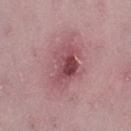No biopsy was performed on this lesion — it was imaged during a full skin examination and was not determined to be concerning. A male subject, about 50 years old. A 15 mm crop from a total-body photograph taken for skin-cancer surveillance. From the left thigh. An algorithmic analysis of the crop reported a footprint of about 17 mm², an eccentricity of roughly 0.7, and a shape-asymmetry score of about 0.25 (0 = symmetric). And it measured a lesion-to-skin contrast of about 7.5 (normalized; higher = more distinct). It also reported an automated nevus-likeness rating near 5 out of 100 and lesion-presence confidence of about 100/100. The recorded lesion diameter is about 5.5 mm. Imaged with white-light lighting.